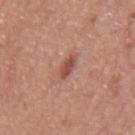image source — ~15 mm tile from a whole-body skin photo | body site — the mid back | lighting — white-light | patient — male, aged 73–77.